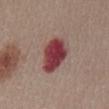biopsy status — catalogued during a skin exam; not biopsied
patient — female, aged 28–32
site — the abdomen
lesion diameter — about 5 mm
image — 15 mm crop, total-body photography
lighting — white-light
TBP lesion metrics — border irregularity of about 2.5 on a 0–10 scale, a within-lesion color-variation index near 4/10, and peripheral color asymmetry of about 1; a classifier nevus-likeness of about 0/100 and a detector confidence of about 100 out of 100 that the crop contains a lesion